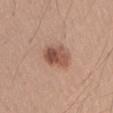<record>
<patient>
  <sex>male</sex>
  <age_approx>20</age_approx>
</patient>
<site>right upper arm</site>
<image>
  <source>total-body photography crop</source>
  <field_of_view_mm>15</field_of_view_mm>
</image>
<automated_metrics>
  <area_mm2_approx>8.0</area_mm2_approx>
  <eccentricity>0.85</eccentricity>
  <shape_asymmetry>0.2</shape_asymmetry>
  <color_variation_0_10>6.5</color_variation_0_10>
  <peripheral_color_asymmetry>2.5</peripheral_color_asymmetry>
</automated_metrics>
<lesion_size>
  <long_diameter_mm_approx>4.0</long_diameter_mm_approx>
</lesion_size>
</record>